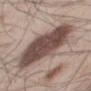notes: no biopsy performed (imaged during a skin exam) | diameter: ~9.5 mm (longest diameter) | automated metrics: a lesion color around L≈47 a*≈16 b*≈20 in CIELAB, roughly 17 lightness units darker than nearby skin, and a normalized border contrast of about 12; a border-irregularity rating of about 2.5/10, a color-variation rating of about 4/10, and peripheral color asymmetry of about 1.5 | lighting: white-light | patient: male, aged 53–57 | location: the mid back | imaging modality: ~15 mm crop, total-body skin-cancer survey.